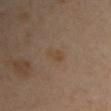No biopsy was performed on this lesion — it was imaged during a full skin examination and was not determined to be concerning.
Captured under cross-polarized illumination.
Measured at roughly 2.5 mm in maximum diameter.
An algorithmic analysis of the crop reported an average lesion color of about L≈45 a*≈15 b*≈30 (CIELAB). And it measured a within-lesion color-variation index near 1/10 and peripheral color asymmetry of about 0. And it measured an automated nevus-likeness rating near 0 out of 100.
A 15 mm close-up tile from a total-body photography series done for melanoma screening.
The patient is a female in their 40s.
On the left upper arm.